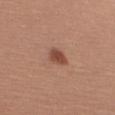<record>
<biopsy_status>not biopsied; imaged during a skin examination</biopsy_status>
<patient>
  <sex>female</sex>
  <age_approx>25</age_approx>
</patient>
<site>right upper arm</site>
<lighting>white-light</lighting>
<lesion_size>
  <long_diameter_mm_approx>2.5</long_diameter_mm_approx>
</lesion_size>
<image>
  <source>total-body photography crop</source>
  <field_of_view_mm>15</field_of_view_mm>
</image>
</record>